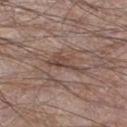No biopsy was performed on this lesion — it was imaged during a full skin examination and was not determined to be concerning. A 15 mm close-up tile from a total-body photography series done for melanoma screening. An algorithmic analysis of the crop reported an average lesion color of about L≈44 a*≈16 b*≈23 (CIELAB) and a normalized border contrast of about 7. And it measured an automated nevus-likeness rating near 0 out of 100. The lesion is on the left thigh. About 4 mm across. The patient is a male aged approximately 55. Imaged with white-light lighting.